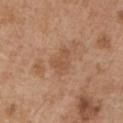Assessment: Recorded during total-body skin imaging; not selected for excision or biopsy. Background: From the left upper arm. A male subject about 55 years old. A lesion tile, about 15 mm wide, cut from a 3D total-body photograph.